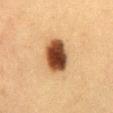Clinical impression: This lesion was catalogued during total-body skin photography and was not selected for biopsy. Image and clinical context: A female patient, aged around 40. Located on the front of the torso. A lesion tile, about 15 mm wide, cut from a 3D total-body photograph.